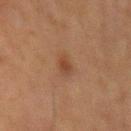{
  "biopsy_status": "not biopsied; imaged during a skin examination",
  "lesion_size": {
    "long_diameter_mm_approx": 2.5
  },
  "patient": {
    "sex": "male",
    "age_approx": 75
  },
  "lighting": "cross-polarized",
  "automated_metrics": {
    "border_irregularity_0_10": 2.0,
    "color_variation_0_10": 1.5,
    "peripheral_color_asymmetry": 0.5
  },
  "image": {
    "source": "total-body photography crop",
    "field_of_view_mm": 15
  },
  "site": "left upper arm"
}